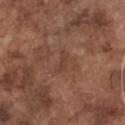| key | value |
|---|---|
| follow-up | imaged on a skin check; not biopsied |
| anatomic site | the chest |
| image | ~15 mm crop, total-body skin-cancer survey |
| subject | male, in their mid-70s |
| tile lighting | white-light |
| diameter | about 2.5 mm |